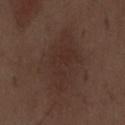Imaged during a routine full-body skin examination; the lesion was not biopsied and no histopathology is available.
The patient is a male aged 68 to 72.
A 15 mm crop from a total-body photograph taken for skin-cancer surveillance.
The lesion is located on the abdomen.